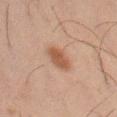No biopsy was performed on this lesion — it was imaged during a full skin examination and was not determined to be concerning. Located on the left thigh. The lesion-visualizer software estimated lesion-presence confidence of about 100/100. The subject is a male aged around 45. Captured under cross-polarized illumination. A 15 mm close-up tile from a total-body photography series done for melanoma screening.